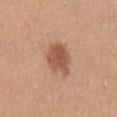{
  "biopsy_status": "not biopsied; imaged during a skin examination",
  "site": "mid back",
  "image": {
    "source": "total-body photography crop",
    "field_of_view_mm": 15
  },
  "lighting": "white-light",
  "automated_metrics": {
    "area_mm2_approx": 9.0,
    "eccentricity": 0.7,
    "shape_asymmetry": 0.3,
    "border_irregularity_0_10": 3.0,
    "color_variation_0_10": 2.5,
    "nevus_likeness_0_100": 95
  },
  "lesion_size": {
    "long_diameter_mm_approx": 4.0
  },
  "patient": {
    "sex": "female",
    "age_approx": 30
  }
}